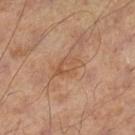| key | value |
|---|---|
| notes | total-body-photography surveillance lesion; no biopsy |
| lighting | cross-polarized illumination |
| diameter | ≈3.5 mm |
| image source | ~15 mm crop, total-body skin-cancer survey |
| location | the left lower leg |
| subject | male, aged approximately 55 |